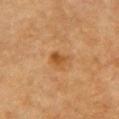The lesion was photographed on a routine skin check and not biopsied; there is no pathology result. Automated tile analysis of the lesion measured a border-irregularity index near 2.5/10 and a peripheral color-asymmetry measure near 1. It also reported lesion-presence confidence of about 100/100. This image is a 15 mm lesion crop taken from a total-body photograph. From the chest. Longest diameter approximately 2.5 mm. The tile uses cross-polarized illumination. The patient is a male roughly 85 years of age or older.